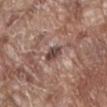Captured during whole-body skin photography for melanoma surveillance; the lesion was not biopsied.